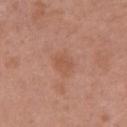biopsy_status: not biopsied; imaged during a skin examination